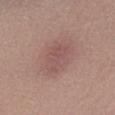Assessment: Recorded during total-body skin imaging; not selected for excision or biopsy. Image and clinical context: This image is a 15 mm lesion crop taken from a total-body photograph. The recorded lesion diameter is about 4.5 mm. A female subject aged approximately 55. On the right lower leg.